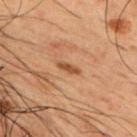- biopsy status — imaged on a skin check; not biopsied
- diameter — ≈2.5 mm
- location — the chest
- patient — male, roughly 50 years of age
- acquisition — 15 mm crop, total-body photography